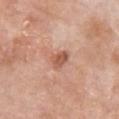Clinical impression:
Part of a total-body skin-imaging series; this lesion was reviewed on a skin check and was not flagged for biopsy.
Background:
The lesion-visualizer software estimated a lesion area of about 4.5 mm², an outline eccentricity of about 0.7 (0 = round, 1 = elongated), and a symmetry-axis asymmetry near 0.25. And it measured a mean CIELAB color near L≈56 a*≈24 b*≈31, a lesion–skin lightness drop of about 11, and a normalized lesion–skin contrast near 7. The patient is a female aged 73 to 77. This image is a 15 mm lesion crop taken from a total-body photograph. The lesion is located on the front of the torso. The tile uses white-light illumination.